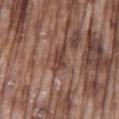Clinical summary: A region of skin cropped from a whole-body photographic capture, roughly 15 mm wide. A male subject roughly 75 years of age. On the mid back.